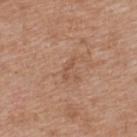The lesion was photographed on a routine skin check and not biopsied; there is no pathology result.
The lesion's longest dimension is about 3 mm.
A female subject, aged around 40.
Captured under white-light illumination.
On the upper back.
A close-up tile cropped from a whole-body skin photograph, about 15 mm across.